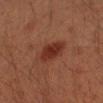workup: no biopsy performed (imaged during a skin exam) | image-analysis metrics: an average lesion color of about L≈26 a*≈22 b*≈24 (CIELAB), a lesion–skin lightness drop of about 8, and a normalized border contrast of about 8.5; a nevus-likeness score of about 95/100 and lesion-presence confidence of about 100/100 | subject: male, aged 43–47 | location: the right upper arm | imaging modality: 15 mm crop, total-body photography | lighting: cross-polarized.